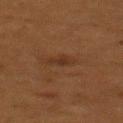No biopsy was performed on this lesion — it was imaged during a full skin examination and was not determined to be concerning. A female subject, about 50 years old. The tile uses cross-polarized illumination. The lesion is located on the upper back. Longest diameter approximately 3 mm. A lesion tile, about 15 mm wide, cut from a 3D total-body photograph. An algorithmic analysis of the crop reported a border-irregularity index near 4/10, a color-variation rating of about 1.5/10, and radial color variation of about 0.5. The analysis additionally found a nevus-likeness score of about 0/100 and a lesion-detection confidence of about 100/100.